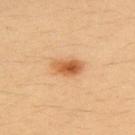Q: Automated lesion metrics?
A: a classifier nevus-likeness of about 100/100
Q: How was the tile lit?
A: cross-polarized
Q: What is the lesion's diameter?
A: ≈3.5 mm
Q: Where on the body is the lesion?
A: the back
Q: What is the imaging modality?
A: ~15 mm tile from a whole-body skin photo
Q: What are the patient's age and sex?
A: female, in their mid-30s